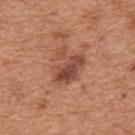Part of a total-body skin-imaging series; this lesion was reviewed on a skin check and was not flagged for biopsy.
Cropped from a whole-body photographic skin survey; the tile spans about 15 mm.
The tile uses white-light illumination.
A male patient, in their mid-60s.
The lesion-visualizer software estimated an average lesion color of about L≈48 a*≈25 b*≈30 (CIELAB) and a lesion-to-skin contrast of about 8 (normalized; higher = more distinct). And it measured a within-lesion color-variation index near 6.5/10 and a peripheral color-asymmetry measure near 2.5. The analysis additionally found a classifier nevus-likeness of about 65/100 and lesion-presence confidence of about 100/100.
Located on the mid back.